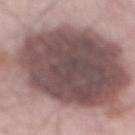<record>
<biopsy_status>not biopsied; imaged during a skin examination</biopsy_status>
<lighting>white-light</lighting>
<site>back</site>
<patient>
  <sex>male</sex>
  <age_approx>65</age_approx>
</patient>
<automated_metrics>
  <eccentricity>0.75</eccentricity>
  <shape_asymmetry>0.35</shape_asymmetry>
  <cielab_L>50</cielab_L>
  <cielab_a>17</cielab_a>
  <cielab_b>18</cielab_b>
  <vs_skin_darker_L>17.0</vs_skin_darker_L>
  <vs_skin_contrast_norm>11.5</vs_skin_contrast_norm>
  <color_variation_0_10>7.0</color_variation_0_10>
  <peripheral_color_asymmetry>2.0</peripheral_color_asymmetry>
  <lesion_detection_confidence_0_100>85</lesion_detection_confidence_0_100>
</automated_metrics>
<image>
  <source>total-body photography crop</source>
  <field_of_view_mm>15</field_of_view_mm>
</image>
</record>